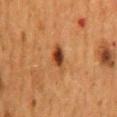Assessment:
No biopsy was performed on this lesion — it was imaged during a full skin examination and was not determined to be concerning.
Image and clinical context:
On the mid back. A female patient aged around 50. This image is a 15 mm lesion crop taken from a total-body photograph. The lesion's longest dimension is about 4 mm. Captured under cross-polarized illumination.